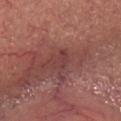workup — imaged on a skin check; not biopsied
image-analysis metrics — a lesion color around L≈40 a*≈25 b*≈21 in CIELAB, about 5 CIELAB-L* units darker than the surrounding skin, and a normalized border contrast of about 4.5; border irregularity of about 5 on a 0–10 scale, a color-variation rating of about 1.5/10, and a peripheral color-asymmetry measure near 0.5
anatomic site — the head or neck
patient — male, aged around 60
imaging modality — 15 mm crop, total-body photography